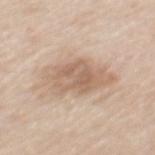Clinical impression: Part of a total-body skin-imaging series; this lesion was reviewed on a skin check and was not flagged for biopsy. Image and clinical context: From the mid back. About 7 mm across. An algorithmic analysis of the crop reported an area of roughly 20 mm², an eccentricity of roughly 0.8, and a symmetry-axis asymmetry near 0.2. The analysis additionally found a lesion–skin lightness drop of about 10 and a normalized lesion–skin contrast near 6.5. It also reported border irregularity of about 3 on a 0–10 scale, internal color variation of about 4 on a 0–10 scale, and radial color variation of about 1.5. The software also gave a classifier nevus-likeness of about 20/100 and a lesion-detection confidence of about 100/100. A male subject in their mid-60s. A roughly 15 mm field-of-view crop from a total-body skin photograph.